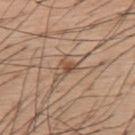imaging modality = 15 mm crop, total-body photography; patient = male, roughly 55 years of age; anatomic site = the upper back.